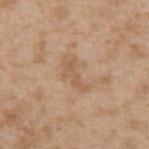Recorded during total-body skin imaging; not selected for excision or biopsy. Imaged with white-light lighting. The lesion-visualizer software estimated a border-irregularity rating of about 7.5/10, a color-variation rating of about 1/10, and peripheral color asymmetry of about 0. Located on the left upper arm. Approximately 4 mm at its widest. A close-up tile cropped from a whole-body skin photograph, about 15 mm across. A male patient about 45 years old.